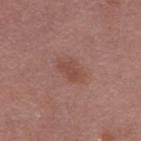workup: no biopsy performed (imaged during a skin exam) | patient: female, aged 38–42 | lighting: white-light illumination | image-analysis metrics: an area of roughly 3.5 mm², a shape eccentricity near 0.85, and a symmetry-axis asymmetry near 0.3; border irregularity of about 3 on a 0–10 scale, a color-variation rating of about 1/10, and radial color variation of about 0 | anatomic site: the left thigh | lesion size: ≈2.5 mm | acquisition: total-body-photography crop, ~15 mm field of view.